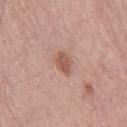<tbp_lesion>
  <biopsy_status>not biopsied; imaged during a skin examination</biopsy_status>
  <site>leg</site>
  <patient>
    <sex>male</sex>
    <age_approx>75</age_approx>
  </patient>
  <lighting>white-light</lighting>
  <lesion_size>
    <long_diameter_mm_approx>3.0</long_diameter_mm_approx>
  </lesion_size>
  <image>
    <source>total-body photography crop</source>
    <field_of_view_mm>15</field_of_view_mm>
  </image>
</tbp_lesion>